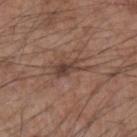This image is a 15 mm lesion crop taken from a total-body photograph.
A male subject roughly 55 years of age.
On the left forearm.